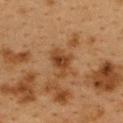The tile uses cross-polarized illumination. A female patient aged around 40. A lesion tile, about 15 mm wide, cut from a 3D total-body photograph. Approximately 3.5 mm at its widest. The lesion is on the back. The total-body-photography lesion software estimated a color-variation rating of about 3.5/10 and radial color variation of about 1. It also reported a nevus-likeness score of about 15/100 and a lesion-detection confidence of about 100/100.